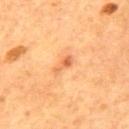* workup — no biopsy performed (imaged during a skin exam)
* acquisition — ~15 mm crop, total-body skin-cancer survey
* lesion size — ≈3 mm
* automated metrics — a footprint of about 3 mm², an outline eccentricity of about 0.9 (0 = round, 1 = elongated), and two-axis asymmetry of about 0.35; a border-irregularity index near 3.5/10, a within-lesion color-variation index near 2/10, and a peripheral color-asymmetry measure near 0.5
* location — the mid back
* subject — male, aged approximately 55
* lighting — cross-polarized